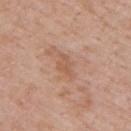Assessment: No biopsy was performed on this lesion — it was imaged during a full skin examination and was not determined to be concerning. Acquisition and patient details: The subject is a male aged 68 to 72. This image is a 15 mm lesion crop taken from a total-body photograph. The lesion is located on the upper back.